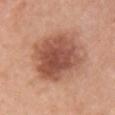Imaged during a routine full-body skin examination; the lesion was not biopsied and no histopathology is available. Captured under white-light illumination. A close-up tile cropped from a whole-body skin photograph, about 15 mm across. Measured at roughly 6.5 mm in maximum diameter. A female patient roughly 60 years of age. The lesion is on the right upper arm.